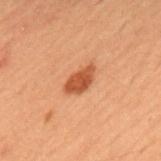Case summary:
- workup · no biopsy performed (imaged during a skin exam)
- diameter · ≈4 mm
- subject · male, about 50 years old
- automated metrics · border irregularity of about 2.5 on a 0–10 scale and peripheral color asymmetry of about 0.5; a detector confidence of about 100 out of 100 that the crop contains a lesion
- illumination · cross-polarized
- image source · ~15 mm tile from a whole-body skin photo
- site · the upper back